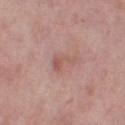Imaged during a routine full-body skin examination; the lesion was not biopsied and no histopathology is available. A 15 mm close-up extracted from a 3D total-body photography capture. A female patient roughly 50 years of age. Imaged with white-light lighting. An algorithmic analysis of the crop reported a shape eccentricity near 0.9 and a shape-asymmetry score of about 0.5 (0 = symmetric). It also reported a lesion color around L≈56 a*≈22 b*≈24 in CIELAB and a normalized lesion–skin contrast near 5. The analysis additionally found a nevus-likeness score of about 0/100 and a lesion-detection confidence of about 100/100. On the right thigh.